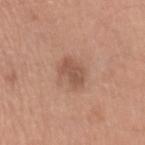Impression:
Captured during whole-body skin photography for melanoma surveillance; the lesion was not biopsied.
Acquisition and patient details:
The total-body-photography lesion software estimated about 10 CIELAB-L* units darker than the surrounding skin and a normalized lesion–skin contrast near 6.5. And it measured lesion-presence confidence of about 100/100. The patient is a male approximately 30 years of age. Measured at roughly 3.5 mm in maximum diameter. A 15 mm crop from a total-body photograph taken for skin-cancer surveillance. On the left upper arm.